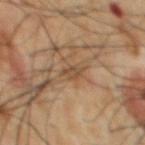The lesion was tiled from a total-body skin photograph and was not biopsied. Cropped from a total-body skin-imaging series; the visible field is about 15 mm. Imaged with cross-polarized lighting. On the chest. The subject is a male roughly 65 years of age.